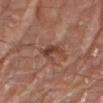<tbp_lesion>
  <biopsy_status>not biopsied; imaged during a skin examination</biopsy_status>
  <image>
    <source>total-body photography crop</source>
    <field_of_view_mm>15</field_of_view_mm>
  </image>
  <site>right forearm</site>
  <automated_metrics>
    <lesion_detection_confidence_0_100>100</lesion_detection_confidence_0_100>
  </automated_metrics>
  <patient>
    <sex>male</sex>
    <age_approx>80</age_approx>
  </patient>
  <lesion_size>
    <long_diameter_mm_approx>3.0</long_diameter_mm_approx>
  </lesion_size>
  <lighting>white-light</lighting>
</tbp_lesion>